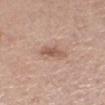No biopsy was performed on this lesion — it was imaged during a full skin examination and was not determined to be concerning.
The lesion is on the leg.
A female subject roughly 65 years of age.
This image is a 15 mm lesion crop taken from a total-body photograph.
The lesion's longest dimension is about 3.5 mm.
An algorithmic analysis of the crop reported an area of roughly 5 mm², an eccentricity of roughly 0.85, and a symmetry-axis asymmetry near 0.35. The software also gave a mean CIELAB color near L≈57 a*≈19 b*≈28 and about 10 CIELAB-L* units darker than the surrounding skin.